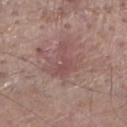• body site · the right lower leg
• lesion size · about 4 mm
• imaging modality · ~15 mm tile from a whole-body skin photo
• lighting · white-light
• subject · male, aged approximately 80